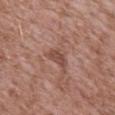Imaged during a routine full-body skin examination; the lesion was not biopsied and no histopathology is available. This is a white-light tile. The lesion's longest dimension is about 2.5 mm. A male subject, about 45 years old. On the mid back. A 15 mm close-up tile from a total-body photography series done for melanoma screening.